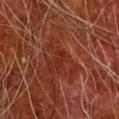biopsy_status: not biopsied; imaged during a skin examination
lighting: cross-polarized
site: right forearm
image:
  source: total-body photography crop
  field_of_view_mm: 15
patient:
  sex: male
  age_approx: 80